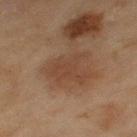Clinical impression: The lesion was tiled from a total-body skin photograph and was not biopsied. Acquisition and patient details: On the left thigh. Captured under cross-polarized illumination. The patient is a female roughly 60 years of age. Automated tile analysis of the lesion measured a lesion area of about 12 mm² and an outline eccentricity of about 0.65 (0 = round, 1 = elongated). And it measured a detector confidence of about 100 out of 100 that the crop contains a lesion. A roughly 15 mm field-of-view crop from a total-body skin photograph. About 5 mm across.